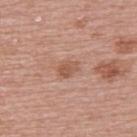follow-up: no biopsy performed (imaged during a skin exam)
imaging modality: 15 mm crop, total-body photography
subject: female, aged 58–62
TBP lesion metrics: an average lesion color of about L≈55 a*≈22 b*≈30 (CIELAB), roughly 9 lightness units darker than nearby skin, and a normalized border contrast of about 6.5; a border-irregularity index near 2/10 and a color-variation rating of about 2.5/10; an automated nevus-likeness rating near 20 out of 100 and lesion-presence confidence of about 100/100
anatomic site: the upper back